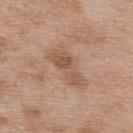- notes: total-body-photography surveillance lesion; no biopsy
- image-analysis metrics: a lesion area of about 9.5 mm²; border irregularity of about 3.5 on a 0–10 scale, a color-variation rating of about 4.5/10, and peripheral color asymmetry of about 1.5; an automated nevus-likeness rating near 0 out of 100
- illumination: white-light
- lesion diameter: ≈5 mm
- anatomic site: the upper back
- patient: male, aged around 50
- image: total-body-photography crop, ~15 mm field of view